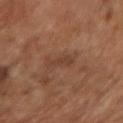The lesion was tiled from a total-body skin photograph and was not biopsied.
This image is a 15 mm lesion crop taken from a total-body photograph.
The lesion's longest dimension is about 3 mm.
A female subject, approximately 65 years of age.
On the right forearm.
This is a cross-polarized tile.